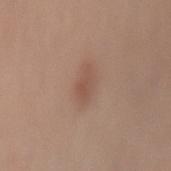Notes:
– biopsy status — imaged on a skin check; not biopsied
– location — the mid back
– size — ≈3.5 mm
– illumination — white-light illumination
– patient — male, about 40 years old
– imaging modality — ~15 mm crop, total-body skin-cancer survey